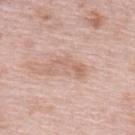Assessment:
Imaged during a routine full-body skin examination; the lesion was not biopsied and no histopathology is available.
Image and clinical context:
Approximately 4.5 mm at its widest. Imaged with white-light lighting. A lesion tile, about 15 mm wide, cut from a 3D total-body photograph. The subject is a female in their mid-60s. On the upper back.